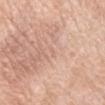biopsy status — imaged on a skin check; not biopsied | subject — female, in their mid-50s | image-analysis metrics — a footprint of about 1 mm², an eccentricity of roughly 0.95, and two-axis asymmetry of about 0.35 | site — the arm | acquisition — 15 mm crop, total-body photography | lesion diameter — about 2 mm.